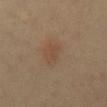Part of a total-body skin-imaging series; this lesion was reviewed on a skin check and was not flagged for biopsy.
This image is a 15 mm lesion crop taken from a total-body photograph.
From the mid back.
A female subject, approximately 60 years of age.